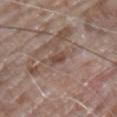follow-up: imaged on a skin check; not biopsied | anatomic site: the left upper arm | image: ~15 mm tile from a whole-body skin photo | subject: male, aged approximately 65.